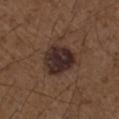Imaged during a routine full-body skin examination; the lesion was not biopsied and no histopathology is available. The patient is a male approximately 50 years of age. Automated image analysis of the tile measured a footprint of about 15 mm² and a shape-asymmetry score of about 0.25 (0 = symmetric). It also reported a border-irregularity rating of about 2.5/10, a color-variation rating of about 5/10, and peripheral color asymmetry of about 1.5. Imaged with white-light lighting. A 15 mm close-up tile from a total-body photography series done for melanoma screening. Located on the left upper arm.